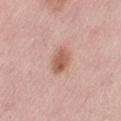Case summary:
– follow-up · catalogued during a skin exam; not biopsied
– illumination · white-light illumination
– lesion size · about 3.5 mm
– imaging modality · 15 mm crop, total-body photography
– patient · female, aged 48 to 52
– body site · the front of the torso